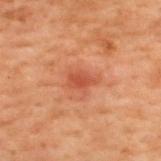Part of a total-body skin-imaging series; this lesion was reviewed on a skin check and was not flagged for biopsy. On the upper back. This is a cross-polarized tile. About 2.5 mm across. A male subject, about 70 years old. A region of skin cropped from a whole-body photographic capture, roughly 15 mm wide.